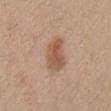This image is a 15 mm lesion crop taken from a total-body photograph. The tile uses white-light illumination. A male patient aged 28 to 32. From the abdomen. The total-body-photography lesion software estimated a shape eccentricity near 0.85 and a shape-asymmetry score of about 0.2 (0 = symmetric). The software also gave a border-irregularity rating of about 2.5/10 and internal color variation of about 3 on a 0–10 scale. The analysis additionally found a detector confidence of about 100 out of 100 that the crop contains a lesion.